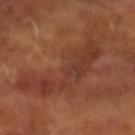  biopsy_status: not biopsied; imaged during a skin examination
  lighting: cross-polarized
  patient:
    sex: male
    age_approx: 65
  image:
    source: total-body photography crop
    field_of_view_mm: 15
  lesion_size:
    long_diameter_mm_approx: 11.0